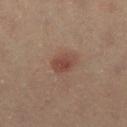Q: Was a biopsy performed?
A: catalogued during a skin exam; not biopsied
Q: Lesion location?
A: the right lower leg
Q: How was this image acquired?
A: total-body-photography crop, ~15 mm field of view
Q: What is the lesion's diameter?
A: about 2.5 mm
Q: How was the tile lit?
A: cross-polarized illumination
Q: Patient demographics?
A: female, aged 48–52